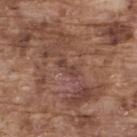notes — total-body-photography surveillance lesion; no biopsy | automated metrics — an area of roughly 2.5 mm²; a lesion–skin lightness drop of about 7 and a lesion-to-skin contrast of about 6.5 (normalized; higher = more distinct); a classifier nevus-likeness of about 0/100 and a lesion-detection confidence of about 80/100 | location — the upper back | illumination — white-light | image — 15 mm crop, total-body photography | subject — male, approximately 75 years of age | diameter — ~2.5 mm (longest diameter).